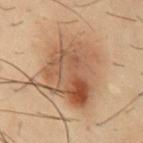| key | value |
|---|---|
| follow-up | catalogued during a skin exam; not biopsied |
| site | the mid back |
| acquisition | ~15 mm crop, total-body skin-cancer survey |
| patient | male, in their 30s |